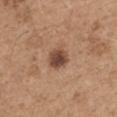biopsy status = total-body-photography surveillance lesion; no biopsy | size = about 3 mm | tile lighting = white-light | subject = male, aged 63–67 | image = ~15 mm tile from a whole-body skin photo | site = the chest | automated lesion analysis = an area of roughly 6.5 mm², an eccentricity of roughly 0.55, and two-axis asymmetry of about 0.2; a border-irregularity index near 2/10; a nevus-likeness score of about 80/100.